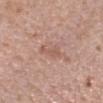Clinical impression: Imaged during a routine full-body skin examination; the lesion was not biopsied and no histopathology is available. Background: The lesion's longest dimension is about 3 mm. From the right forearm. The tile uses white-light illumination. The lesion-visualizer software estimated an average lesion color of about L≈56 a*≈21 b*≈27 (CIELAB), about 7 CIELAB-L* units darker than the surrounding skin, and a normalized lesion–skin contrast near 5. A female subject aged 58 to 62. A 15 mm crop from a total-body photograph taken for skin-cancer surveillance.